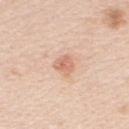Case summary:
– biopsy status: no biopsy performed (imaged during a skin exam)
– size: ≈3 mm
– image: total-body-photography crop, ~15 mm field of view
– TBP lesion metrics: a lesion area of about 5 mm², an outline eccentricity of about 0.55 (0 = round, 1 = elongated), and a shape-asymmetry score of about 0.3 (0 = symmetric); a classifier nevus-likeness of about 30/100 and lesion-presence confidence of about 100/100
– subject: female, about 45 years old
– anatomic site: the chest
– lighting: white-light illumination